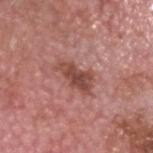This lesion was catalogued during total-body skin photography and was not selected for biopsy. A close-up tile cropped from a whole-body skin photograph, about 15 mm across. The lesion is located on the head or neck. A male subject aged 73–77. About 4.5 mm across. Captured under white-light illumination.